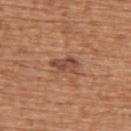Captured during whole-body skin photography for melanoma surveillance; the lesion was not biopsied. Imaged with white-light lighting. The lesion is on the back. The patient is a female aged 63–67. The lesion-visualizer software estimated a footprint of about 5 mm², an eccentricity of roughly 0.85, and a shape-asymmetry score of about 0.3 (0 = symmetric). The analysis additionally found a lesion color around L≈47 a*≈23 b*≈30 in CIELAB and about 10 CIELAB-L* units darker than the surrounding skin. The analysis additionally found a border-irregularity index near 3/10, internal color variation of about 3 on a 0–10 scale, and peripheral color asymmetry of about 1. The software also gave a classifier nevus-likeness of about 50/100 and lesion-presence confidence of about 100/100. A roughly 15 mm field-of-view crop from a total-body skin photograph. About 3.5 mm across.